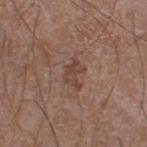{"biopsy_status": "not biopsied; imaged during a skin examination", "lighting": "white-light", "automated_metrics": {"area_mm2_approx": 5.0, "eccentricity": 0.8, "shape_asymmetry": 0.45, "cielab_L": 43, "cielab_a": 19, "cielab_b": 25, "vs_skin_darker_L": 8.0, "border_irregularity_0_10": 5.0, "color_variation_0_10": 2.5, "peripheral_color_asymmetry": 1.0}, "patient": {"sex": "male", "age_approx": 60}, "image": {"source": "total-body photography crop", "field_of_view_mm": 15}, "site": "right lower leg", "lesion_size": {"long_diameter_mm_approx": 3.5}}